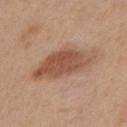Case summary:
• workup: imaged on a skin check; not biopsied
• image: total-body-photography crop, ~15 mm field of view
• TBP lesion metrics: a border-irregularity index near 3/10, internal color variation of about 4 on a 0–10 scale, and peripheral color asymmetry of about 1.5; a nevus-likeness score of about 70/100
• illumination: white-light
• lesion diameter: about 8 mm
• patient: male, aged 28–32
• body site: the front of the torso